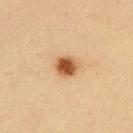  biopsy_status: not biopsied; imaged during a skin examination
  image:
    source: total-body photography crop
    field_of_view_mm: 15
  site: upper back
  automated_metrics:
    eccentricity: 0.4
    shape_asymmetry: 0.1
    border_irregularity_0_10: 1.0
    color_variation_0_10: 3.5
    peripheral_color_asymmetry: 1.0
    nevus_likeness_0_100: 100
    lesion_detection_confidence_0_100: 100
  lighting: cross-polarized
  lesion_size:
    long_diameter_mm_approx: 2.5
  patient:
    sex: male
    age_approx: 35